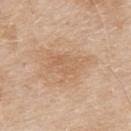Captured during whole-body skin photography for melanoma surveillance; the lesion was not biopsied. The patient is a male aged approximately 80. On the upper back. The lesion's longest dimension is about 5.5 mm. A 15 mm close-up extracted from a 3D total-body photography capture. Imaged with white-light lighting.